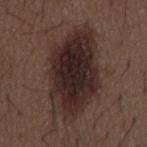notes: imaged on a skin check; not biopsied | lighting: white-light illumination | size: about 11 mm | image source: 15 mm crop, total-body photography | subject: male, aged around 50 | body site: the mid back.